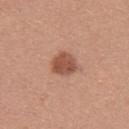The lesion was photographed on a routine skin check and not biopsied; there is no pathology result. Captured under white-light illumination. About 3.5 mm across. A female subject, in their mid-30s. On the upper back. Cropped from a whole-body photographic skin survey; the tile spans about 15 mm.